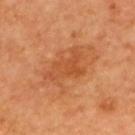Captured during whole-body skin photography for melanoma surveillance; the lesion was not biopsied. A male patient, about 65 years old. On the upper back. Cropped from a whole-body photographic skin survey; the tile spans about 15 mm. Longest diameter approximately 5 mm. This is a cross-polarized tile.